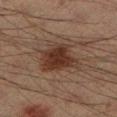The lesion was tiled from a total-body skin photograph and was not biopsied.
Cropped from a whole-body photographic skin survey; the tile spans about 15 mm.
A male subject, in their 40s.
The lesion is located on the left lower leg.